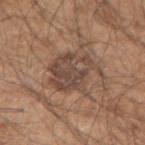The lesion was photographed on a routine skin check and not biopsied; there is no pathology result.
The tile uses white-light illumination.
The lesion is on the right upper arm.
The lesion-visualizer software estimated an outline eccentricity of about 0.35 (0 = round, 1 = elongated) and a symmetry-axis asymmetry near 0.4. It also reported border irregularity of about 5.5 on a 0–10 scale and peripheral color asymmetry of about 1.5. It also reported an automated nevus-likeness rating near 20 out of 100 and a lesion-detection confidence of about 75/100.
A roughly 15 mm field-of-view crop from a total-body skin photograph.
Approximately 6 mm at its widest.
The patient is a male about 50 years old.